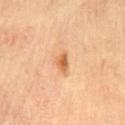<record>
<biopsy_status>not biopsied; imaged during a skin examination</biopsy_status>
<site>lower back</site>
<image>
  <source>total-body photography crop</source>
  <field_of_view_mm>15</field_of_view_mm>
</image>
<patient>
  <sex>male</sex>
  <age_approx>70</age_approx>
</patient>
<lighting>cross-polarized</lighting>
<lesion_size>
  <long_diameter_mm_approx>2.5</long_diameter_mm_approx>
</lesion_size>
</record>